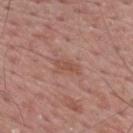workup: total-body-photography surveillance lesion; no biopsy
image-analysis metrics: a lesion area of about 4 mm², a shape eccentricity near 0.9, and two-axis asymmetry of about 0.4; border irregularity of about 4.5 on a 0–10 scale, a color-variation rating of about 1.5/10, and radial color variation of about 0.5
lighting: white-light
size: about 3 mm
image: ~15 mm tile from a whole-body skin photo
location: the back
patient: male, aged 53 to 57